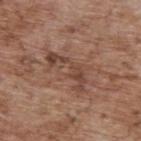On the back.
Imaged with white-light lighting.
About 5.5 mm across.
The total-body-photography lesion software estimated a border-irregularity rating of about 10/10, a within-lesion color-variation index near 2.5/10, and peripheral color asymmetry of about 0.5.
A 15 mm close-up extracted from a 3D total-body photography capture.
The subject is a male approximately 70 years of age.